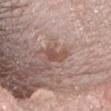Recorded during total-body skin imaging; not selected for excision or biopsy. A 15 mm close-up tile from a total-body photography series done for melanoma screening. A male subject in their 60s. The total-body-photography lesion software estimated a shape eccentricity near 0.85 and two-axis asymmetry of about 0.4. It also reported a lesion color around L≈51 a*≈20 b*≈26 in CIELAB, a lesion–skin lightness drop of about 9, and a normalized border contrast of about 7.5. The lesion's longest dimension is about 3 mm. From the head or neck.